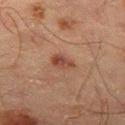{
  "biopsy_status": "not biopsied; imaged during a skin examination",
  "site": "right thigh",
  "automated_metrics": {
    "area_mm2_approx": 4.0,
    "eccentricity": 0.8,
    "shape_asymmetry": 0.3,
    "cielab_L": 35,
    "cielab_a": 20,
    "cielab_b": 24,
    "vs_skin_darker_L": 8.0,
    "vs_skin_contrast_norm": 7.5
  },
  "lesion_size": {
    "long_diameter_mm_approx": 3.0
  },
  "lighting": "cross-polarized",
  "patient": {
    "sex": "male",
    "age_approx": 65
  },
  "image": {
    "source": "total-body photography crop",
    "field_of_view_mm": 15
  }
}